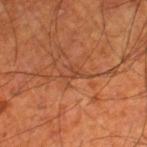<case>
  <lighting>cross-polarized</lighting>
  <site>left thigh</site>
  <automated_metrics>
    <area_mm2_approx>2.5</area_mm2_approx>
    <eccentricity>0.8</eccentricity>
    <shape_asymmetry>0.5</shape_asymmetry>
    <cielab_L>43</cielab_L>
    <cielab_a>26</cielab_a>
    <cielab_b>34</cielab_b>
    <vs_skin_darker_L>6.0</vs_skin_darker_L>
    <vs_skin_contrast_norm>5.0</vs_skin_contrast_norm>
    <lesion_detection_confidence_0_100>55</lesion_detection_confidence_0_100>
  </automated_metrics>
  <patient>
    <sex>male</sex>
    <age_approx>70</age_approx>
  </patient>
  <image>
    <source>total-body photography crop</source>
    <field_of_view_mm>15</field_of_view_mm>
  </image>
</case>